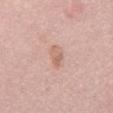This lesion was catalogued during total-body skin photography and was not selected for biopsy. Located on the mid back. A female subject, aged 63–67. This image is a 15 mm lesion crop taken from a total-body photograph.